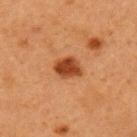Impression:
Part of a total-body skin-imaging series; this lesion was reviewed on a skin check and was not flagged for biopsy.
Context:
A female subject, aged around 40. Cropped from a total-body skin-imaging series; the visible field is about 15 mm. On the left upper arm.